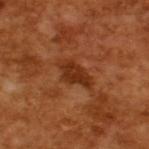Clinical impression: The lesion was tiled from a total-body skin photograph and was not biopsied. Background: Approximately 4 mm at its widest. A male subject, aged approximately 65. Cropped from a whole-body photographic skin survey; the tile spans about 15 mm.